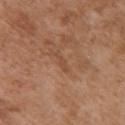Q: Was this lesion biopsied?
A: imaged on a skin check; not biopsied
Q: How large is the lesion?
A: ~2.5 mm (longest diameter)
Q: Lesion location?
A: the right upper arm
Q: What lighting was used for the tile?
A: white-light
Q: What are the patient's age and sex?
A: male, about 75 years old
Q: How was this image acquired?
A: ~15 mm crop, total-body skin-cancer survey